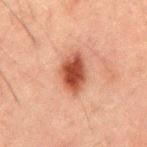The subject is a male aged 48 to 52. A 15 mm crop from a total-body photograph taken for skin-cancer surveillance. From the mid back. Automated image analysis of the tile measured an area of roughly 10 mm², an eccentricity of roughly 0.75, and two-axis asymmetry of about 0.15. It also reported a border-irregularity rating of about 2/10 and a peripheral color-asymmetry measure near 1. The analysis additionally found an automated nevus-likeness rating near 100 out of 100 and a lesion-detection confidence of about 100/100.